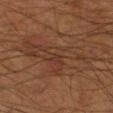Findings:
* biopsy status · total-body-photography surveillance lesion; no biopsy
* image · 15 mm crop, total-body photography
* anatomic site · the right lower leg
* tile lighting · cross-polarized
* patient · male, about 55 years old